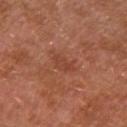Captured during whole-body skin photography for melanoma surveillance; the lesion was not biopsied. Cropped from a total-body skin-imaging series; the visible field is about 15 mm. The subject is a male about 30 years old. The tile uses cross-polarized illumination. About 2.5 mm across. The lesion is on the left upper arm.